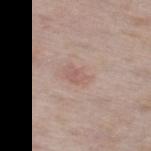A roughly 15 mm field-of-view crop from a total-body skin photograph. A female subject about 60 years old. On the right thigh.